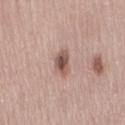| field | value |
|---|---|
| notes | catalogued during a skin exam; not biopsied |
| lighting | white-light illumination |
| subject | female, in their 50s |
| lesion size | about 3.5 mm |
| location | the front of the torso |
| imaging modality | 15 mm crop, total-body photography |